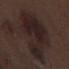Impression: The lesion was photographed on a routine skin check and not biopsied; there is no pathology result. Acquisition and patient details: An algorithmic analysis of the crop reported an automated nevus-likeness rating near 50 out of 100 and lesion-presence confidence of about 95/100. Cropped from a whole-body photographic skin survey; the tile spans about 15 mm. The subject is a male in their 70s. The tile uses white-light illumination. Located on the right lower leg. About 12 mm across.